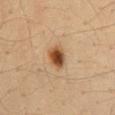workup: no biopsy performed (imaged during a skin exam) | diameter: ~3 mm (longest diameter) | acquisition: total-body-photography crop, ~15 mm field of view | location: the mid back | image-analysis metrics: about 15 CIELAB-L* units darker than the surrounding skin and a normalized lesion–skin contrast near 11; a border-irregularity rating of about 1.5/10, a color-variation rating of about 6/10, and peripheral color asymmetry of about 1.5; a nevus-likeness score of about 100/100 and a detector confidence of about 100 out of 100 that the crop contains a lesion | tile lighting: cross-polarized illumination | subject: male, in their mid-30s.